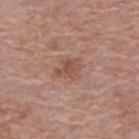Recorded during total-body skin imaging; not selected for excision or biopsy. The patient is a female aged approximately 65. The total-body-photography lesion software estimated a nevus-likeness score of about 5/100 and a lesion-detection confidence of about 100/100. Cropped from a whole-body photographic skin survey; the tile spans about 15 mm. The lesion is on the back. Captured under white-light illumination. Measured at roughly 3 mm in maximum diameter.